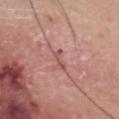Q: Illumination type?
A: white-light illumination
Q: How large is the lesion?
A: ≈2.5 mm
Q: What is the anatomic site?
A: the head or neck
Q: Patient demographics?
A: male, about 65 years old
Q: Automated lesion metrics?
A: radial color variation of about 0
Q: What kind of image is this?
A: total-body-photography crop, ~15 mm field of view